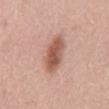Approximately 5 mm at its widest.
An algorithmic analysis of the crop reported a lesion–skin lightness drop of about 13 and a normalized lesion–skin contrast near 9. The software also gave a border-irregularity index near 2.5/10 and a within-lesion color-variation index near 4.5/10. The software also gave an automated nevus-likeness rating near 95 out of 100 and lesion-presence confidence of about 100/100.
Imaged with white-light lighting.
A male patient, roughly 50 years of age.
The lesion is on the mid back.
A close-up tile cropped from a whole-body skin photograph, about 15 mm across.